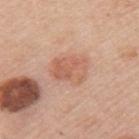{
  "biopsy_status": "not biopsied; imaged during a skin examination"
}